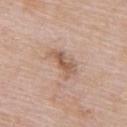notes: imaged on a skin check; not biopsied.